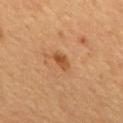notes=no biopsy performed (imaged during a skin exam)
tile lighting=cross-polarized illumination
image source=total-body-photography crop, ~15 mm field of view
patient=male, aged 53 to 57
lesion diameter=≈2.5 mm
site=the mid back
image-analysis metrics=a footprint of about 3.5 mm², an eccentricity of roughly 0.8, and a symmetry-axis asymmetry near 0.3; border irregularity of about 2.5 on a 0–10 scale, internal color variation of about 3.5 on a 0–10 scale, and peripheral color asymmetry of about 1